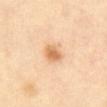workup: imaged on a skin check; not biopsied
image-analysis metrics: a lesion color around L≈70 a*≈22 b*≈41 in CIELAB, a lesion–skin lightness drop of about 12, and a lesion-to-skin contrast of about 8 (normalized; higher = more distinct); a border-irregularity index near 2/10, internal color variation of about 3.5 on a 0–10 scale, and a peripheral color-asymmetry measure near 1; a classifier nevus-likeness of about 95/100 and a lesion-detection confidence of about 100/100
image: total-body-photography crop, ~15 mm field of view
patient: female, roughly 60 years of age
diameter: ~2.5 mm (longest diameter)
lighting: cross-polarized illumination
body site: the abdomen